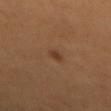Captured during whole-body skin photography for melanoma surveillance; the lesion was not biopsied. A male patient, aged 48 to 52. Approximately 2 mm at its widest. The lesion-visualizer software estimated a footprint of about 2.5 mm², an eccentricity of roughly 0.8, and two-axis asymmetry of about 0.25. It also reported a normalized border contrast of about 6.5. The software also gave an automated nevus-likeness rating near 95 out of 100 and a detector confidence of about 100 out of 100 that the crop contains a lesion. From the mid back. A 15 mm crop from a total-body photograph taken for skin-cancer surveillance.